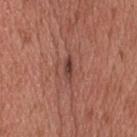Located on the head or neck.
A male patient aged 53 to 57.
The lesion's longest dimension is about 2.5 mm.
Imaged with white-light lighting.
A 15 mm close-up tile from a total-body photography series done for melanoma screening.